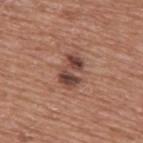{"biopsy_status": "not biopsied; imaged during a skin examination", "patient": {"sex": "male", "age_approx": 75}, "image": {"source": "total-body photography crop", "field_of_view_mm": 15}, "automated_metrics": {"cielab_L": 44, "cielab_a": 21, "cielab_b": 25, "vs_skin_darker_L": 12.0, "vs_skin_contrast_norm": 9.5}, "site": "upper back", "lesion_size": {"long_diameter_mm_approx": 4.0}, "lighting": "white-light"}